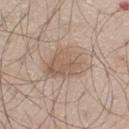Captured during whole-body skin photography for melanoma surveillance; the lesion was not biopsied. From the left thigh. Automated image analysis of the tile measured an average lesion color of about L≈58 a*≈14 b*≈27 (CIELAB). And it measured a nevus-likeness score of about 10/100 and lesion-presence confidence of about 100/100. A 15 mm close-up extracted from a 3D total-body photography capture. A male patient aged around 60. This is a white-light tile.